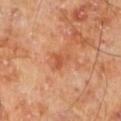follow-up: imaged on a skin check; not biopsied
imaging modality: ~15 mm tile from a whole-body skin photo
diameter: ≈3 mm
tile lighting: cross-polarized illumination
patient: male, aged 68 to 72
location: the left lower leg